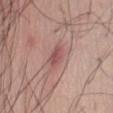Q: Was this lesion biopsied?
A: catalogued during a skin exam; not biopsied
Q: Lesion location?
A: the abdomen
Q: Automated lesion metrics?
A: a lesion area of about 5 mm² and an eccentricity of roughly 0.8; a border-irregularity index near 1.5/10 and a within-lesion color-variation index near 2.5/10
Q: Who is the patient?
A: male, aged approximately 35
Q: What is the imaging modality?
A: ~15 mm crop, total-body skin-cancer survey
Q: Illumination type?
A: white-light